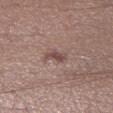The lesion was photographed on a routine skin check and not biopsied; there is no pathology result.
On the leg.
A lesion tile, about 15 mm wide, cut from a 3D total-body photograph.
A male subject, about 55 years old.